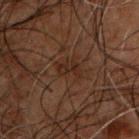workup = catalogued during a skin exam; not biopsied
subject = male, approximately 50 years of age
lesion diameter = ~3 mm (longest diameter)
TBP lesion metrics = a lesion area of about 3.5 mm² and a shape eccentricity near 0.9; a mean CIELAB color near L≈19 a*≈15 b*≈20 and a normalized lesion–skin contrast near 6.5; a border-irregularity index near 4.5/10, internal color variation of about 0 on a 0–10 scale, and peripheral color asymmetry of about 0
location = the chest
acquisition = 15 mm crop, total-body photography